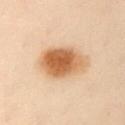{"biopsy_status": "not biopsied; imaged during a skin examination", "patient": {"sex": "female", "age_approx": 60}, "site": "chest", "lesion_size": {"long_diameter_mm_approx": 5.5}, "image": {"source": "total-body photography crop", "field_of_view_mm": 15}, "lighting": "cross-polarized"}